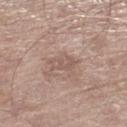Assessment: No biopsy was performed on this lesion — it was imaged during a full skin examination and was not determined to be concerning. Context: On the leg. Automated tile analysis of the lesion measured a nevus-likeness score of about 0/100 and a lesion-detection confidence of about 100/100. The lesion's longest dimension is about 2.5 mm. A roughly 15 mm field-of-view crop from a total-body skin photograph. Captured under white-light illumination. A male subject, approximately 70 years of age.